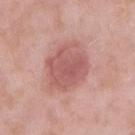Part of a total-body skin-imaging series; this lesion was reviewed on a skin check and was not flagged for biopsy.
This is a white-light tile.
Cropped from a total-body skin-imaging series; the visible field is about 15 mm.
Measured at roughly 5.5 mm in maximum diameter.
A male patient aged around 55.
On the right upper arm.